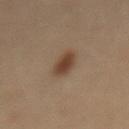biopsy_status: not biopsied; imaged during a skin examination
site: mid back
lighting: cross-polarized
image:
  source: total-body photography crop
  field_of_view_mm: 15
patient:
  sex: male
  age_approx: 60
lesion_size:
  long_diameter_mm_approx: 3.5
automated_metrics:
  area_mm2_approx: 6.0
  eccentricity: 0.8
  shape_asymmetry: 0.15
  cielab_L: 40
  cielab_a: 16
  cielab_b: 27
  vs_skin_contrast_norm: 9.0
  border_irregularity_0_10: 1.5
  color_variation_0_10: 2.5
  peripheral_color_asymmetry: 1.0
  nevus_likeness_0_100: 100
  lesion_detection_confidence_0_100: 100